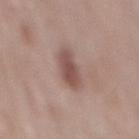– biopsy status — no biopsy performed (imaged during a skin exam)
– imaging modality — ~15 mm tile from a whole-body skin photo
– site — the back
– subject — male, aged approximately 55
– lighting — white-light illumination